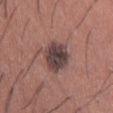The lesion was tiled from a total-body skin photograph and was not biopsied. The subject is a male aged 43 to 47. Captured under white-light illumination. The lesion is on the right thigh. Measured at roughly 4.5 mm in maximum diameter. A lesion tile, about 15 mm wide, cut from a 3D total-body photograph. Automated image analysis of the tile measured border irregularity of about 1.5 on a 0–10 scale, internal color variation of about 4.5 on a 0–10 scale, and peripheral color asymmetry of about 1.5. And it measured a nevus-likeness score of about 55/100 and lesion-presence confidence of about 100/100.